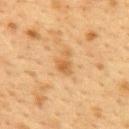| feature | finding |
|---|---|
| workup | imaged on a skin check; not biopsied |
| size | ~3 mm (longest diameter) |
| body site | the upper back |
| subject | female, aged 38–42 |
| acquisition | ~15 mm tile from a whole-body skin photo |